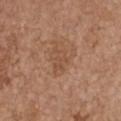Case summary:
* notes — catalogued during a skin exam; not biopsied
* image source — total-body-photography crop, ~15 mm field of view
* subject — female, aged 63–67
* body site — the chest